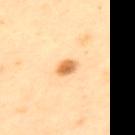Assessment:
This lesion was catalogued during total-body skin photography and was not selected for biopsy.
Background:
From the upper back. A female patient aged around 45. About 3 mm across. This image is a 15 mm lesion crop taken from a total-body photograph. The tile uses cross-polarized illumination.